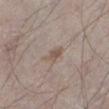Captured during whole-body skin photography for melanoma surveillance; the lesion was not biopsied. A lesion tile, about 15 mm wide, cut from a 3D total-body photograph. The subject is a male aged 73–77. On the left lower leg.